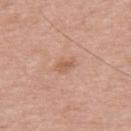Assessment: No biopsy was performed on this lesion — it was imaged during a full skin examination and was not determined to be concerning. Acquisition and patient details: Longest diameter approximately 2.5 mm. This is a white-light tile. A male patient approximately 55 years of age. A roughly 15 mm field-of-view crop from a total-body skin photograph.